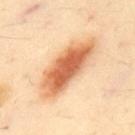biopsy status: catalogued during a skin exam; not biopsied.